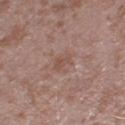Recorded during total-body skin imaging; not selected for excision or biopsy. The subject is a male aged 43 to 47. Automated tile analysis of the lesion measured an area of roughly 3.5 mm² and a shape-asymmetry score of about 0.4 (0 = symmetric). It also reported a lesion color around L≈51 a*≈19 b*≈24 in CIELAB. And it measured internal color variation of about 1.5 on a 0–10 scale and radial color variation of about 0.5. The analysis additionally found lesion-presence confidence of about 100/100. A 15 mm close-up tile from a total-body photography series done for melanoma screening. Measured at roughly 2.5 mm in maximum diameter. From the right thigh. This is a white-light tile.